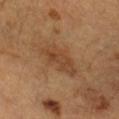  biopsy_status: not biopsied; imaged during a skin examination
  site: head or neck
  lighting: cross-polarized
  image:
    source: total-body photography crop
    field_of_view_mm: 15
  lesion_size:
    long_diameter_mm_approx: 4.5
  patient:
    sex: male
    age_approx: 65
  automated_metrics:
    lesion_detection_confidence_0_100: 100